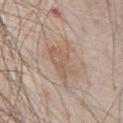No biopsy was performed on this lesion — it was imaged during a full skin examination and was not determined to be concerning.
On the chest.
A roughly 15 mm field-of-view crop from a total-body skin photograph.
A male patient, about 80 years old.